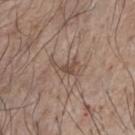Notes:
- notes · imaged on a skin check; not biopsied
- patient · male, approximately 75 years of age
- body site · the chest
- diameter · ≈3.5 mm
- image source · 15 mm crop, total-body photography
- illumination · white-light
- TBP lesion metrics · an average lesion color of about L≈49 a*≈16 b*≈25 (CIELAB) and roughly 8 lightness units darker than nearby skin; a classifier nevus-likeness of about 0/100 and a detector confidence of about 95 out of 100 that the crop contains a lesion